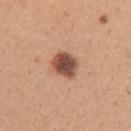Findings:
* workup — imaged on a skin check; not biopsied
* image source — ~15 mm crop, total-body skin-cancer survey
* patient — female, aged approximately 30
* body site — the right upper arm